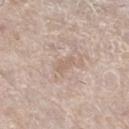  biopsy_status: not biopsied; imaged during a skin examination
  site: left lower leg
  lighting: white-light
  patient:
    sex: female
    age_approx: 75
  image:
    source: total-body photography crop
    field_of_view_mm: 15